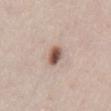Assessment:
The lesion was tiled from a total-body skin photograph and was not biopsied.
Image and clinical context:
A roughly 15 mm field-of-view crop from a total-body skin photograph. An algorithmic analysis of the crop reported a footprint of about 4.5 mm², a shape eccentricity near 0.7, and two-axis asymmetry of about 0.2. It also reported a lesion color around L≈54 a*≈19 b*≈26 in CIELAB and a lesion-to-skin contrast of about 11 (normalized; higher = more distinct). The patient is a male in their 40s. The tile uses white-light illumination. Located on the abdomen.